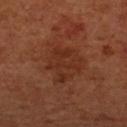No biopsy was performed on this lesion — it was imaged during a full skin examination and was not determined to be concerning.
A female subject, in their mid-60s.
The lesion's longest dimension is about 5 mm.
The lesion-visualizer software estimated an outline eccentricity of about 0.35 (0 = round, 1 = elongated) and two-axis asymmetry of about 0.3. It also reported a classifier nevus-likeness of about 0/100 and a detector confidence of about 100 out of 100 that the crop contains a lesion.
Cropped from a total-body skin-imaging series; the visible field is about 15 mm.
Imaged with cross-polarized lighting.
Located on the left forearm.